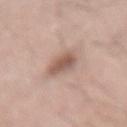Q: What kind of image is this?
A: total-body-photography crop, ~15 mm field of view
Q: Where on the body is the lesion?
A: the arm
Q: What lighting was used for the tile?
A: white-light illumination
Q: Patient demographics?
A: male, aged 58–62
Q: What did automated image analysis measure?
A: an area of roughly 7 mm², an outline eccentricity of about 0.6 (0 = round, 1 = elongated), and a symmetry-axis asymmetry near 0.25; a border-irregularity rating of about 2.5/10, a color-variation rating of about 3.5/10, and a peripheral color-asymmetry measure near 1; a classifier nevus-likeness of about 70/100 and a lesion-detection confidence of about 100/100
Q: Lesion size?
A: ~3 mm (longest diameter)